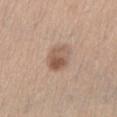The lesion was photographed on a routine skin check and not biopsied; there is no pathology result. The total-body-photography lesion software estimated a lesion area of about 8 mm². It also reported a mean CIELAB color near L≈56 a*≈17 b*≈28, a lesion–skin lightness drop of about 11, and a normalized lesion–skin contrast near 7.5. The analysis additionally found a border-irregularity rating of about 1.5/10 and radial color variation of about 3. Captured under white-light illumination. Measured at roughly 4 mm in maximum diameter. A female patient about 45 years old. A 15 mm crop from a total-body photograph taken for skin-cancer surveillance. The lesion is located on the abdomen.